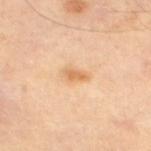Clinical impression:
Imaged during a routine full-body skin examination; the lesion was not biopsied and no histopathology is available.
Context:
A 15 mm close-up extracted from a 3D total-body photography capture. Longest diameter approximately 2.5 mm. Captured under cross-polarized illumination. The total-body-photography lesion software estimated an area of roughly 3.5 mm² and a symmetry-axis asymmetry near 0.3. The software also gave a lesion color around L≈68 a*≈21 b*≈41 in CIELAB, about 9 CIELAB-L* units darker than the surrounding skin, and a lesion-to-skin contrast of about 7 (normalized; higher = more distinct). The software also gave border irregularity of about 2.5 on a 0–10 scale, a color-variation rating of about 1/10, and a peripheral color-asymmetry measure near 0.5. A patient aged 53 to 57. The lesion is on the left thigh.